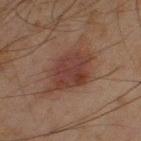<lesion>
  <biopsy_status>not biopsied; imaged during a skin examination</biopsy_status>
  <lighting>cross-polarized</lighting>
  <patient>
    <sex>male</sex>
    <age_approx>45</age_approx>
  </patient>
  <site>left thigh</site>
  <lesion_size>
    <long_diameter_mm_approx>6.0</long_diameter_mm_approx>
  </lesion_size>
  <automated_metrics>
    <vs_skin_darker_L>8.0</vs_skin_darker_L>
    <vs_skin_contrast_norm>7.5</vs_skin_contrast_norm>
    <border_irregularity_0_10>3.0</border_irregularity_0_10>
    <color_variation_0_10>3.0</color_variation_0_10>
    <peripheral_color_asymmetry>1.0</peripheral_color_asymmetry>
  </automated_metrics>
  <image>
    <source>total-body photography crop</source>
    <field_of_view_mm>15</field_of_view_mm>
  </image>
</lesion>